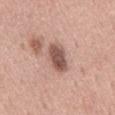biopsy status: catalogued during a skin exam; not biopsied
image: 15 mm crop, total-body photography
location: the mid back
automated lesion analysis: a shape eccentricity near 0.85 and a shape-asymmetry score of about 0.2 (0 = symmetric); a border-irregularity rating of about 2.5/10 and a color-variation rating of about 4/10; an automated nevus-likeness rating near 25 out of 100 and lesion-presence confidence of about 100/100
lesion size: ≈4 mm
tile lighting: white-light
patient: male, about 55 years old